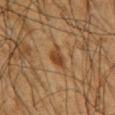Case summary:
– follow-up · no biopsy performed (imaged during a skin exam)